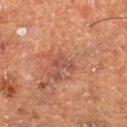Assessment: Captured during whole-body skin photography for melanoma surveillance; the lesion was not biopsied. Background: This is a cross-polarized tile. A lesion tile, about 15 mm wide, cut from a 3D total-body photograph. The patient is a male aged 73 to 77. The lesion is located on the right thigh. The lesion-visualizer software estimated a shape-asymmetry score of about 0.35 (0 = symmetric). And it measured border irregularity of about 3.5 on a 0–10 scale and a within-lesion color-variation index near 1/10. The analysis additionally found a nevus-likeness score of about 0/100 and a lesion-detection confidence of about 100/100. Approximately 2.5 mm at its widest.